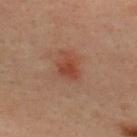workup = catalogued during a skin exam; not biopsied
diameter = ≈3 mm
illumination = cross-polarized illumination
imaging modality = ~15 mm tile from a whole-body skin photo
anatomic site = the upper back
subject = male, roughly 35 years of age
TBP lesion metrics = a footprint of about 5 mm², a shape eccentricity near 0.65, and two-axis asymmetry of about 0.35; an average lesion color of about L≈42 a*≈25 b*≈30 (CIELAB); a border-irregularity rating of about 3/10, a within-lesion color-variation index near 2/10, and peripheral color asymmetry of about 0.5; a nevus-likeness score of about 75/100 and a detector confidence of about 100 out of 100 that the crop contains a lesion